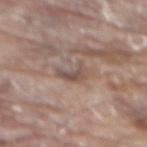Notes:
• follow-up: no biopsy performed (imaged during a skin exam)
• anatomic site: the back
• TBP lesion metrics: a lesion area of about 4.5 mm², an eccentricity of roughly 0.75, and a symmetry-axis asymmetry near 0.45; an average lesion color of about L≈51 a*≈15 b*≈22 (CIELAB); border irregularity of about 4.5 on a 0–10 scale, a within-lesion color-variation index near 2.5/10, and peripheral color asymmetry of about 0.5; a classifier nevus-likeness of about 0/100 and a lesion-detection confidence of about 75/100
• lighting: white-light illumination
• lesion size: ≈3 mm
• acquisition: 15 mm crop, total-body photography
• patient: male, aged around 80